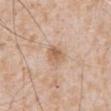image source: ~15 mm crop, total-body skin-cancer survey
patient: male, about 65 years old
body site: the abdomen